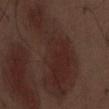Part of a total-body skin-imaging series; this lesion was reviewed on a skin check and was not flagged for biopsy.
This is a white-light tile.
Located on the abdomen.
A male subject, aged 68 to 72.
The recorded lesion diameter is about 11.5 mm.
This image is a 15 mm lesion crop taken from a total-body photograph.